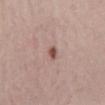Assessment:
Imaged during a routine full-body skin examination; the lesion was not biopsied and no histopathology is available.
Background:
This is a white-light tile. A 15 mm close-up extracted from a 3D total-body photography capture. An algorithmic analysis of the crop reported about 13 CIELAB-L* units darker than the surrounding skin. The software also gave a border-irregularity rating of about 1.5/10, a within-lesion color-variation index near 1.5/10, and radial color variation of about 0.5. The software also gave a classifier nevus-likeness of about 90/100 and a lesion-detection confidence of about 100/100. The recorded lesion diameter is about 1.5 mm. A female subject, aged 18 to 22. The lesion is located on the leg.